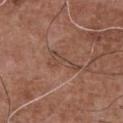Recorded during total-body skin imaging; not selected for excision or biopsy. On the front of the torso. Approximately 4.5 mm at its widest. Captured under white-light illumination. A 15 mm close-up tile from a total-body photography series done for melanoma screening. A male patient aged 73 to 77.